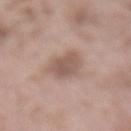Part of a total-body skin-imaging series; this lesion was reviewed on a skin check and was not flagged for biopsy. Cropped from a total-body skin-imaging series; the visible field is about 15 mm. Located on the lower back. A male patient, aged around 55.